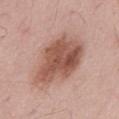Assessment:
Imaged during a routine full-body skin examination; the lesion was not biopsied and no histopathology is available.
Context:
A male subject roughly 55 years of age. Longest diameter approximately 7 mm. The lesion is located on the mid back. Captured under white-light illumination. The total-body-photography lesion software estimated a nevus-likeness score of about 40/100 and a detector confidence of about 100 out of 100 that the crop contains a lesion. A lesion tile, about 15 mm wide, cut from a 3D total-body photograph.